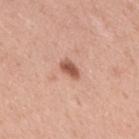Q: Is there a histopathology result?
A: total-body-photography surveillance lesion; no biopsy
Q: Illumination type?
A: white-light
Q: Automated lesion metrics?
A: an average lesion color of about L≈56 a*≈25 b*≈30 (CIELAB), about 14 CIELAB-L* units darker than the surrounding skin, and a normalized border contrast of about 9; a border-irregularity index near 2/10, a within-lesion color-variation index near 2.5/10, and peripheral color asymmetry of about 1; an automated nevus-likeness rating near 95 out of 100 and a lesion-detection confidence of about 100/100
Q: Where on the body is the lesion?
A: the upper back
Q: Who is the patient?
A: male, aged around 50
Q: How was this image acquired?
A: ~15 mm crop, total-body skin-cancer survey